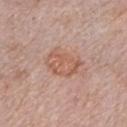Impression:
Part of a total-body skin-imaging series; this lesion was reviewed on a skin check and was not flagged for biopsy.
Image and clinical context:
Longest diameter approximately 4.5 mm. A 15 mm close-up tile from a total-body photography series done for melanoma screening. From the right forearm. Captured under white-light illumination. A female patient, in their mid-60s. An algorithmic analysis of the crop reported a border-irregularity index near 3/10, a color-variation rating of about 3.5/10, and a peripheral color-asymmetry measure near 1.5. And it measured a nevus-likeness score of about 50/100 and a lesion-detection confidence of about 100/100.